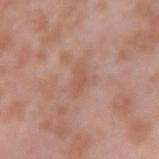  biopsy_status: not biopsied; imaged during a skin examination
  site: right upper arm
  automated_metrics:
    eccentricity: 0.9
    shape_asymmetry: 0.35
    color_variation_0_10: 1.0
    peripheral_color_asymmetry: 0.5
  image:
    source: total-body photography crop
    field_of_view_mm: 15
  patient:
    sex: male
    age_approx: 55
  lighting: white-light
  lesion_size:
    long_diameter_mm_approx: 3.0